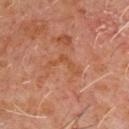No biopsy was performed on this lesion — it was imaged during a full skin examination and was not determined to be concerning. Captured under cross-polarized illumination. Located on the front of the torso. A male patient, aged around 60. The lesion's longest dimension is about 4 mm. A 15 mm close-up extracted from a 3D total-body photography capture.